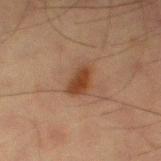Notes:
• notes: catalogued during a skin exam; not biopsied
• patient: male, roughly 65 years of age
• automated lesion analysis: a footprint of about 6 mm², an outline eccentricity of about 0.75 (0 = round, 1 = elongated), and a shape-asymmetry score of about 0.25 (0 = symmetric); a lesion color around L≈34 a*≈18 b*≈27 in CIELAB and about 9 CIELAB-L* units darker than the surrounding skin; a color-variation rating of about 2.5/10 and peripheral color asymmetry of about 1; a classifier nevus-likeness of about 100/100
• image source: ~15 mm crop, total-body skin-cancer survey
• body site: the leg
• tile lighting: cross-polarized